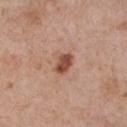The lesion was tiled from a total-body skin photograph and was not biopsied.
A female patient, in their mid-60s.
This image is a 15 mm lesion crop taken from a total-body photograph.
The lesion is on the chest.
Approximately 2.5 mm at its widest.
Automated image analysis of the tile measured an area of roughly 4.5 mm² and a symmetry-axis asymmetry near 0.2. The analysis additionally found border irregularity of about 1.5 on a 0–10 scale, internal color variation of about 2.5 on a 0–10 scale, and peripheral color asymmetry of about 1. And it measured a classifier nevus-likeness of about 95/100 and lesion-presence confidence of about 100/100.